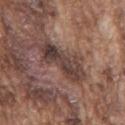The lesion was tiled from a total-body skin photograph and was not biopsied. Located on the mid back. A 15 mm crop from a total-body photograph taken for skin-cancer surveillance. The lesion's longest dimension is about 6 mm. The lesion-visualizer software estimated a lesion area of about 12 mm² and a shape-asymmetry score of about 0.25 (0 = symmetric). It also reported a mean CIELAB color near L≈39 a*≈16 b*≈20, a lesion–skin lightness drop of about 11, and a lesion-to-skin contrast of about 9.5 (normalized; higher = more distinct). The subject is a male roughly 75 years of age.